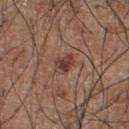<lesion>
  <biopsy_status>not biopsied; imaged during a skin examination</biopsy_status>
  <image>
    <source>total-body photography crop</source>
    <field_of_view_mm>15</field_of_view_mm>
  </image>
  <patient>
    <sex>male</sex>
    <age_approx>55</age_approx>
  </patient>
  <site>chest</site>
  <lighting>white-light</lighting>
</lesion>